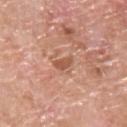Assessment:
No biopsy was performed on this lesion — it was imaged during a full skin examination and was not determined to be concerning.
Context:
A roughly 15 mm field-of-view crop from a total-body skin photograph. Captured under white-light illumination. A male patient, aged around 65. The total-body-photography lesion software estimated a mean CIELAB color near L≈55 a*≈24 b*≈32 and a lesion-to-skin contrast of about 7 (normalized; higher = more distinct). The software also gave a border-irregularity rating of about 3.5/10 and a within-lesion color-variation index near 1/10. The software also gave a classifier nevus-likeness of about 0/100 and a detector confidence of about 100 out of 100 that the crop contains a lesion. Longest diameter approximately 2.5 mm. From the upper back.